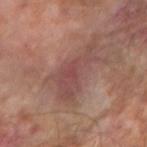A 15 mm close-up tile from a total-body photography series done for melanoma screening. Automated tile analysis of the lesion measured a lesion color around L≈44 a*≈21 b*≈21 in CIELAB and a lesion–skin lightness drop of about 8. The analysis additionally found border irregularity of about 6.5 on a 0–10 scale, a within-lesion color-variation index near 2.5/10, and radial color variation of about 1. Imaged with cross-polarized lighting. Measured at roughly 6.5 mm in maximum diameter. The lesion is on the arm. A male subject, in their mid- to late 60s.